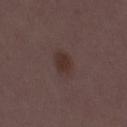Recorded during total-body skin imaging; not selected for excision or biopsy.
A 15 mm close-up extracted from a 3D total-body photography capture.
The subject is a female approximately 30 years of age.
From the right thigh.